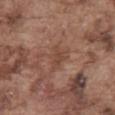biopsy_status: not biopsied; imaged during a skin examination
patient:
  sex: male
  age_approx: 75
site: abdomen
image:
  source: total-body photography crop
  field_of_view_mm: 15